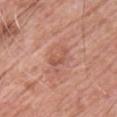workup: no biopsy performed (imaged during a skin exam)
site: the chest
tile lighting: white-light
diameter: about 3 mm
imaging modality: ~15 mm crop, total-body skin-cancer survey
subject: male, roughly 60 years of age
TBP lesion metrics: a footprint of about 3 mm², a shape eccentricity near 0.85, and a shape-asymmetry score of about 0.6 (0 = symmetric); a lesion color around L≈54 a*≈25 b*≈30 in CIELAB, roughly 8 lightness units darker than nearby skin, and a normalized lesion–skin contrast near 5.5; border irregularity of about 7 on a 0–10 scale, internal color variation of about 1 on a 0–10 scale, and a peripheral color-asymmetry measure near 0.5; a classifier nevus-likeness of about 0/100 and a lesion-detection confidence of about 100/100